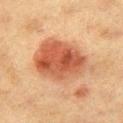  biopsy_status: not biopsied; imaged during a skin examination
  patient:
    sex: female
    age_approx: 40
  image:
    source: total-body photography crop
    field_of_view_mm: 15
  site: left thigh